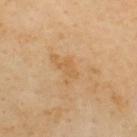{
  "patient": {
    "sex": "male",
    "age_approx": 55
  },
  "lesion_size": {
    "long_diameter_mm_approx": 3.0
  },
  "automated_metrics": {
    "nevus_likeness_0_100": 0,
    "lesion_detection_confidence_0_100": 100
  },
  "image": {
    "source": "total-body photography crop",
    "field_of_view_mm": 15
  },
  "lighting": "cross-polarized",
  "site": "upper back"
}